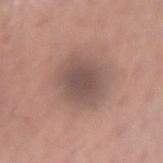Part of a total-body skin-imaging series; this lesion was reviewed on a skin check and was not flagged for biopsy.
This is a white-light tile.
The lesion is located on the right upper arm.
A male patient about 70 years old.
A lesion tile, about 15 mm wide, cut from a 3D total-body photograph.